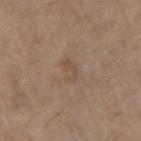Assessment: Imaged during a routine full-body skin examination; the lesion was not biopsied and no histopathology is available. Acquisition and patient details: A roughly 15 mm field-of-view crop from a total-body skin photograph. This is a white-light tile. The patient is a male approximately 65 years of age. The total-body-photography lesion software estimated a lesion area of about 3.5 mm² and two-axis asymmetry of about 0.4. The analysis additionally found a mean CIELAB color near L≈49 a*≈15 b*≈28, a lesion–skin lightness drop of about 6, and a normalized lesion–skin contrast near 4.5. The software also gave a nevus-likeness score of about 0/100 and a detector confidence of about 100 out of 100 that the crop contains a lesion. Located on the left forearm. The recorded lesion diameter is about 2.5 mm.